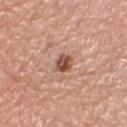Q: Is there a histopathology result?
A: no biopsy performed (imaged during a skin exam)
Q: What lighting was used for the tile?
A: white-light
Q: What is the lesion's diameter?
A: ~2.5 mm (longest diameter)
Q: What is the anatomic site?
A: the right lower leg
Q: How was this image acquired?
A: 15 mm crop, total-body photography
Q: Who is the patient?
A: female, in their mid- to late 60s